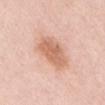Clinical impression: No biopsy was performed on this lesion — it was imaged during a full skin examination and was not determined to be concerning. Context: Imaged with white-light lighting. The lesion is located on the abdomen. The patient is a male about 60 years old. Approximately 5.5 mm at its widest. A lesion tile, about 15 mm wide, cut from a 3D total-body photograph.